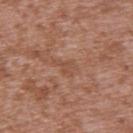Recorded during total-body skin imaging; not selected for excision or biopsy. A roughly 15 mm field-of-view crop from a total-body skin photograph. This is a white-light tile. The lesion is on the back. The patient is a male in their mid-40s. About 3 mm across.